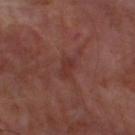biopsy status: imaged on a skin check; not biopsied.